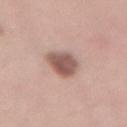| field | value |
|---|---|
| body site | the lower back |
| subject | female, aged around 35 |
| image source | 15 mm crop, total-body photography |
| tile lighting | white-light illumination |
| size | ≈4 mm |